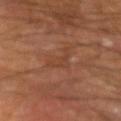Recorded during total-body skin imaging; not selected for excision or biopsy. The lesion is on the left forearm. Longest diameter approximately 4 mm. The total-body-photography lesion software estimated an outline eccentricity of about 0.9 (0 = round, 1 = elongated) and two-axis asymmetry of about 0.6. It also reported a border-irregularity index near 7/10 and a peripheral color-asymmetry measure near 0.5. Captured under cross-polarized illumination. A male patient, in their mid-60s. Cropped from a total-body skin-imaging series; the visible field is about 15 mm.